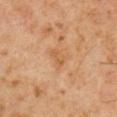The lesion was tiled from a total-body skin photograph and was not biopsied.
This image is a 15 mm lesion crop taken from a total-body photograph.
Automated tile analysis of the lesion measured a mean CIELAB color near L≈54 a*≈21 b*≈37, a lesion–skin lightness drop of about 6, and a normalized lesion–skin contrast near 5.5. It also reported peripheral color asymmetry of about 0.5.
Measured at roughly 2.5 mm in maximum diameter.
Located on the chest.
The subject is a male about 60 years old.